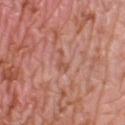Impression:
Imaged during a routine full-body skin examination; the lesion was not biopsied and no histopathology is available.
Acquisition and patient details:
A close-up tile cropped from a whole-body skin photograph, about 15 mm across. The recorded lesion diameter is about 2.5 mm. Located on the right lower leg. The tile uses white-light illumination. Automated image analysis of the tile measured roughly 7 lightness units darker than nearby skin and a normalized lesion–skin contrast near 5.5. And it measured a border-irregularity index near 6.5/10, a color-variation rating of about 0/10, and radial color variation of about 0. The patient is a female about 40 years old.